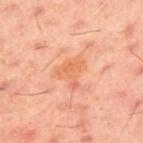Part of a total-body skin-imaging series; this lesion was reviewed on a skin check and was not flagged for biopsy. Longest diameter approximately 3.5 mm. A male subject, aged around 60. On the upper back. This image is a 15 mm lesion crop taken from a total-body photograph.